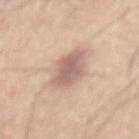Assessment: Recorded during total-body skin imaging; not selected for excision or biopsy. Background: The total-body-photography lesion software estimated an area of roughly 12 mm², an eccentricity of roughly 0.85, and a symmetry-axis asymmetry near 0.25. It also reported a nevus-likeness score of about 35/100 and lesion-presence confidence of about 100/100. A male patient, about 45 years old. A 15 mm close-up tile from a total-body photography series done for melanoma screening. Measured at roughly 5.5 mm in maximum diameter. The lesion is on the mid back.